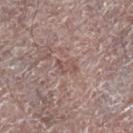Clinical impression:
No biopsy was performed on this lesion — it was imaged during a full skin examination and was not determined to be concerning.
Image and clinical context:
Automated image analysis of the tile measured an area of roughly 3.5 mm² and a shape-asymmetry score of about 0.35 (0 = symmetric). The software also gave a lesion–skin lightness drop of about 7. And it measured border irregularity of about 3.5 on a 0–10 scale, internal color variation of about 1.5 on a 0–10 scale, and peripheral color asymmetry of about 0.5. The analysis additionally found an automated nevus-likeness rating near 0 out of 100 and a lesion-detection confidence of about 85/100. The subject is a male roughly 65 years of age. The lesion is located on the right lower leg. Measured at roughly 2.5 mm in maximum diameter. This image is a 15 mm lesion crop taken from a total-body photograph. Captured under white-light illumination.